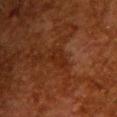biopsy status: no biopsy performed (imaged during a skin exam) | site: the upper back | TBP lesion metrics: an average lesion color of about L≈21 a*≈21 b*≈27 (CIELAB), a lesion–skin lightness drop of about 5, and a normalized border contrast of about 6 | lesion diameter: ~3 mm (longest diameter) | lighting: cross-polarized illumination | image: ~15 mm crop, total-body skin-cancer survey | patient: female, approximately 50 years of age.